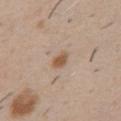follow-up = catalogued during a skin exam; not biopsied | subject = male, aged approximately 50 | imaging modality = 15 mm crop, total-body photography | lesion size = about 2.5 mm | tile lighting = white-light illumination | location = the chest.